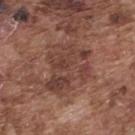Impression: No biopsy was performed on this lesion — it was imaged during a full skin examination and was not determined to be concerning. Background: The lesion is on the upper back. A region of skin cropped from a whole-body photographic capture, roughly 15 mm wide. The subject is a male aged 73–77.